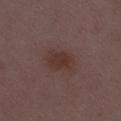  biopsy_status: not biopsied; imaged during a skin examination
  patient:
    sex: female
    age_approx: 30
  site: leg
  image:
    source: total-body photography crop
    field_of_view_mm: 15